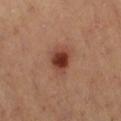notes = total-body-photography surveillance lesion; no biopsy
imaging modality = ~15 mm tile from a whole-body skin photo
body site = the right thigh
patient = female, aged approximately 40